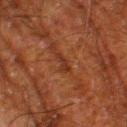| key | value |
|---|---|
| notes | no biopsy performed (imaged during a skin exam) |
| lesion diameter | ≈3 mm |
| lighting | cross-polarized |
| acquisition | total-body-photography crop, ~15 mm field of view |
| patient | male, roughly 80 years of age |
| location | the left thigh |